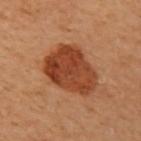- biopsy status: catalogued during a skin exam; not biopsied
- lighting: cross-polarized
- patient: female, aged around 60
- image: 15 mm crop, total-body photography
- lesion diameter: about 6 mm
- automated lesion analysis: an area of roughly 22 mm² and an outline eccentricity of about 0.65 (0 = round, 1 = elongated); a mean CIELAB color near L≈36 a*≈25 b*≈32; border irregularity of about 2.5 on a 0–10 scale and peripheral color asymmetry of about 1.5; a lesion-detection confidence of about 100/100
- anatomic site: the right arm